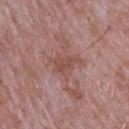Q: Where on the body is the lesion?
A: the upper back
Q: What kind of image is this?
A: ~15 mm crop, total-body skin-cancer survey
Q: Who is the patient?
A: male, aged 63 to 67